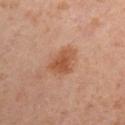No biopsy was performed on this lesion — it was imaged during a full skin examination and was not determined to be concerning.
Measured at roughly 4 mm in maximum diameter.
A male subject, in their mid- to late 40s.
Cropped from a whole-body photographic skin survey; the tile spans about 15 mm.
From the right upper arm.
This is a cross-polarized tile.